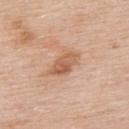follow-up: no biopsy performed (imaged during a skin exam) | lighting: white-light | location: the back | patient: male, about 60 years old | automated lesion analysis: a footprint of about 6.5 mm², a shape eccentricity near 0.85, and a symmetry-axis asymmetry near 0.2; a within-lesion color-variation index near 5/10 and a peripheral color-asymmetry measure near 2 | imaging modality: 15 mm crop, total-body photography.